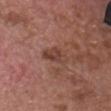Q: Was this lesion biopsied?
A: imaged on a skin check; not biopsied
Q: What lighting was used for the tile?
A: white-light illumination
Q: Lesion location?
A: the chest
Q: What is the imaging modality?
A: total-body-photography crop, ~15 mm field of view
Q: What are the patient's age and sex?
A: male, approximately 75 years of age
Q: Lesion size?
A: ≈3.5 mm
Q: Automated lesion metrics?
A: a nevus-likeness score of about 0/100 and a lesion-detection confidence of about 100/100